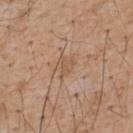This lesion was catalogued during total-body skin photography and was not selected for biopsy. The tile uses white-light illumination. A roughly 15 mm field-of-view crop from a total-body skin photograph. The lesion-visualizer software estimated a mean CIELAB color near L≈55 a*≈18 b*≈32 and a normalized lesion–skin contrast near 5. The analysis additionally found a nevus-likeness score of about 0/100. A male patient roughly 55 years of age. On the upper back.